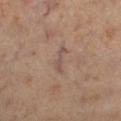The lesion was photographed on a routine skin check and not biopsied; there is no pathology result. This image is a 15 mm lesion crop taken from a total-body photograph. About 3.5 mm across. From the right lower leg. The total-body-photography lesion software estimated a mean CIELAB color near L≈51 a*≈17 b*≈23, about 7 CIELAB-L* units darker than the surrounding skin, and a normalized border contrast of about 5.5. And it measured a nevus-likeness score of about 0/100 and a lesion-detection confidence of about 80/100. A female patient aged approximately 40.